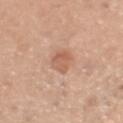follow-up = total-body-photography surveillance lesion; no biopsy
lesion size = about 3 mm
illumination = white-light
imaging modality = ~15 mm tile from a whole-body skin photo
subject = male, about 35 years old
anatomic site = the head or neck